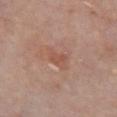| key | value |
|---|---|
| follow-up | no biopsy performed (imaged during a skin exam) |
| patient | female, about 65 years old |
| diameter | about 2 mm |
| tile lighting | white-light |
| imaging modality | ~15 mm crop, total-body skin-cancer survey |
| site | the front of the torso |